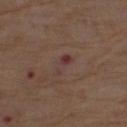Clinical impression: Part of a total-body skin-imaging series; this lesion was reviewed on a skin check and was not flagged for biopsy. Background: A 15 mm close-up tile from a total-body photography series done for melanoma screening. Imaged with white-light lighting. Located on the left thigh. A male patient, roughly 75 years of age.